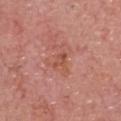Clinical impression: Imaged during a routine full-body skin examination; the lesion was not biopsied and no histopathology is available. Background: A 15 mm crop from a total-body photograph taken for skin-cancer surveillance. The lesion is on the head or neck. A male subject, aged 78–82. About 2.5 mm across.